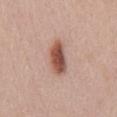biopsy status — no biopsy performed (imaged during a skin exam) | illumination — white-light | patient — female, about 40 years old | size — ≈5 mm | site — the mid back | imaging modality — total-body-photography crop, ~15 mm field of view | automated metrics — border irregularity of about 2.5 on a 0–10 scale, a color-variation rating of about 5/10, and radial color variation of about 1.5; a classifier nevus-likeness of about 100/100 and a detector confidence of about 100 out of 100 that the crop contains a lesion.